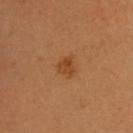Q: Is there a histopathology result?
A: no biopsy performed (imaged during a skin exam)
Q: What did automated image analysis measure?
A: a footprint of about 4 mm², an outline eccentricity of about 0.5 (0 = round, 1 = elongated), and a shape-asymmetry score of about 0.35 (0 = symmetric); a lesion color around L≈44 a*≈24 b*≈39 in CIELAB and about 8 CIELAB-L* units darker than the surrounding skin; border irregularity of about 3 on a 0–10 scale, a color-variation rating of about 3/10, and radial color variation of about 1
Q: What are the patient's age and sex?
A: male, in their 60s
Q: Illumination type?
A: cross-polarized
Q: How was this image acquired?
A: ~15 mm tile from a whole-body skin photo
Q: Lesion size?
A: ≈2.5 mm
Q: What is the anatomic site?
A: the head or neck